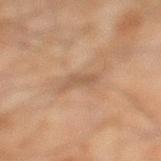The lesion is on the left lower leg. This is a cross-polarized tile. A 15 mm close-up extracted from a 3D total-body photography capture. About 3.5 mm across. The patient is a male approximately 45 years of age.